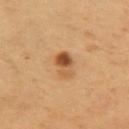workup = total-body-photography surveillance lesion; no biopsy
lesion size = ~3.5 mm (longest diameter)
anatomic site = the arm
subject = male, aged 53 to 57
acquisition = ~15 mm tile from a whole-body skin photo